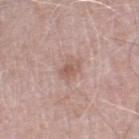Q: Was this lesion biopsied?
A: imaged on a skin check; not biopsied
Q: How large is the lesion?
A: ~2.5 mm (longest diameter)
Q: Where on the body is the lesion?
A: the right thigh
Q: Patient demographics?
A: male, aged 73–77
Q: Automated lesion metrics?
A: an average lesion color of about L≈57 a*≈19 b*≈24 (CIELAB), about 8 CIELAB-L* units darker than the surrounding skin, and a normalized lesion–skin contrast near 6
Q: What kind of image is this?
A: 15 mm crop, total-body photography
Q: How was the tile lit?
A: white-light illumination